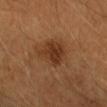<record>
  <biopsy_status>not biopsied; imaged during a skin examination</biopsy_status>
  <patient>
    <sex>male</sex>
    <age_approx>40</age_approx>
  </patient>
  <site>right forearm</site>
  <image>
    <source>total-body photography crop</source>
    <field_of_view_mm>15</field_of_view_mm>
  </image>
  <lighting>cross-polarized</lighting>
  <lesion_size>
    <long_diameter_mm_approx>5.0</long_diameter_mm_approx>
  </lesion_size>
</record>